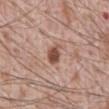biopsy_status: not biopsied; imaged during a skin examination
site: abdomen
image:
  source: total-body photography crop
  field_of_view_mm: 15
lesion_size:
  long_diameter_mm_approx: 3.0
patient:
  sex: male
  age_approx: 70